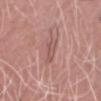workup: total-body-photography surveillance lesion; no biopsy | imaging modality: ~15 mm tile from a whole-body skin photo | illumination: white-light illumination | body site: the chest | subject: male, aged approximately 40 | diameter: about 3.5 mm.